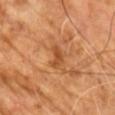This lesion was catalogued during total-body skin photography and was not selected for biopsy. Cropped from a total-body skin-imaging series; the visible field is about 15 mm. From the chest. The recorded lesion diameter is about 3 mm. A male subject, aged 68–72.